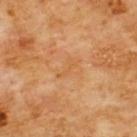Impression: This lesion was catalogued during total-body skin photography and was not selected for biopsy. Image and clinical context: A male patient, in their 60s. Captured under cross-polarized illumination. Measured at roughly 2.5 mm in maximum diameter. A lesion tile, about 15 mm wide, cut from a 3D total-body photograph. Located on the chest.